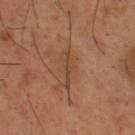{
  "biopsy_status": "not biopsied; imaged during a skin examination",
  "patient": {
    "sex": "male",
    "age_approx": 40
  },
  "lesion_size": {
    "long_diameter_mm_approx": 4.0
  },
  "automated_metrics": {
    "area_mm2_approx": 4.5,
    "eccentricity": 0.95,
    "shape_asymmetry": 0.5,
    "cielab_L": 43,
    "cielab_a": 19,
    "cielab_b": 31,
    "vs_skin_darker_L": 7.0,
    "vs_skin_contrast_norm": 5.5,
    "nevus_likeness_0_100": 0,
    "lesion_detection_confidence_0_100": 90
  },
  "image": {
    "source": "total-body photography crop",
    "field_of_view_mm": 15
  },
  "site": "upper back",
  "lighting": "cross-polarized"
}